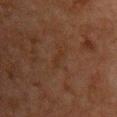Findings:
• follow-up · catalogued during a skin exam; not biopsied
• patient · male, in their 60s
• illumination · cross-polarized illumination
• anatomic site · the chest
• image · 15 mm crop, total-body photography